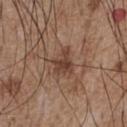| key | value |
|---|---|
| follow-up | no biopsy performed (imaged during a skin exam) |
| subject | male, aged 53 to 57 |
| location | the chest |
| image source | total-body-photography crop, ~15 mm field of view |
| size | ~3 mm (longest diameter) |
| illumination | white-light illumination |